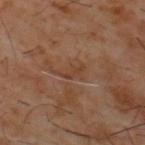– follow-up — imaged on a skin check; not biopsied
– image-analysis metrics — a lesion area of about 2.5 mm² and a shape eccentricity near 0.9; an average lesion color of about L≈37 a*≈19 b*≈27 (CIELAB) and a lesion–skin lightness drop of about 6; a border-irregularity rating of about 6.5/10, a within-lesion color-variation index near 0/10, and peripheral color asymmetry of about 0; a nevus-likeness score of about 0/100 and a lesion-detection confidence of about 95/100
– size — ~3 mm (longest diameter)
– patient — male, about 60 years old
– location — the upper back
– image — ~15 mm tile from a whole-body skin photo
– lighting — cross-polarized